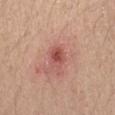Q: Was this lesion biopsied?
A: total-body-photography surveillance lesion; no biopsy
Q: What kind of image is this?
A: 15 mm crop, total-body photography
Q: What are the patient's age and sex?
A: female, aged 43–47
Q: Illumination type?
A: white-light illumination
Q: Automated lesion metrics?
A: a footprint of about 4.5 mm² and an outline eccentricity of about 0.65 (0 = round, 1 = elongated); a nevus-likeness score of about 70/100
Q: Lesion location?
A: the left forearm
Q: What is the lesion's diameter?
A: about 3 mm